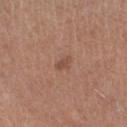{"biopsy_status": "not biopsied; imaged during a skin examination", "image": {"source": "total-body photography crop", "field_of_view_mm": 15}, "patient": {"sex": "female", "age_approx": 45}, "automated_metrics": {"border_irregularity_0_10": 2.5, "color_variation_0_10": 0.5, "nevus_likeness_0_100": 10, "lesion_detection_confidence_0_100": 100}, "lighting": "white-light", "site": "right lower leg"}